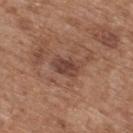{
  "lighting": "white-light",
  "lesion_size": {
    "long_diameter_mm_approx": 3.5
  },
  "image": {
    "source": "total-body photography crop",
    "field_of_view_mm": 15
  },
  "site": "upper back",
  "patient": {
    "sex": "male",
    "age_approx": 65
  }
}